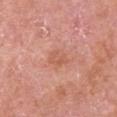The lesion is located on the left upper arm.
A male patient aged around 70.
This image is a 15 mm lesion crop taken from a total-body photograph.